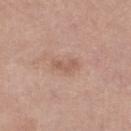follow-up: imaged on a skin check; not biopsied | patient: female, in their mid- to late 60s | body site: the left thigh | diameter: about 3 mm | illumination: white-light | image source: ~15 mm tile from a whole-body skin photo.